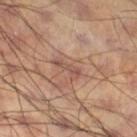notes: imaged on a skin check; not biopsied | illumination: cross-polarized illumination | imaging modality: 15 mm crop, total-body photography | patient: male, aged 58 to 62 | location: the right thigh.